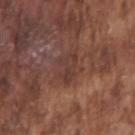Longest diameter approximately 3.5 mm. A 15 mm close-up tile from a total-body photography series done for melanoma screening. The lesion is located on the right upper arm. Imaged with white-light lighting. A male patient aged 73 to 77.